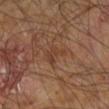Part of a total-body skin-imaging series; this lesion was reviewed on a skin check and was not flagged for biopsy. From the right leg. The total-body-photography lesion software estimated a lesion area of about 4.5 mm² and two-axis asymmetry of about 0.45. The software also gave a mean CIELAB color near L≈38 a*≈19 b*≈28, a lesion–skin lightness drop of about 6, and a normalized border contrast of about 5.5. And it measured a nevus-likeness score of about 0/100 and lesion-presence confidence of about 95/100. A close-up tile cropped from a whole-body skin photograph, about 15 mm across. A male subject roughly 60 years of age. Captured under cross-polarized illumination.